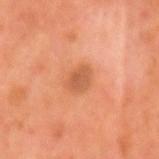Captured during whole-body skin photography for melanoma surveillance; the lesion was not biopsied.
Automated tile analysis of the lesion measured a border-irregularity index near 2/10, a within-lesion color-variation index near 1.5/10, and a peripheral color-asymmetry measure near 0.5. The analysis additionally found an automated nevus-likeness rating near 20 out of 100.
A 15 mm crop from a total-body photograph taken for skin-cancer surveillance.
The subject is a male aged 53–57.
This is a cross-polarized tile.
The lesion is located on the head or neck.